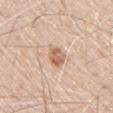| field | value |
|---|---|
| biopsy status | total-body-photography surveillance lesion; no biopsy |
| image source | ~15 mm tile from a whole-body skin photo |
| anatomic site | the back |
| subject | male, approximately 70 years of age |
| lighting | white-light |
| size | ≈3 mm |
| TBP lesion metrics | a lesion area of about 5.5 mm², an eccentricity of roughly 0.75, and a symmetry-axis asymmetry near 0.15; an average lesion color of about L≈64 a*≈19 b*≈32 (CIELAB) and a normalized border contrast of about 8; a nevus-likeness score of about 80/100 and lesion-presence confidence of about 100/100 |